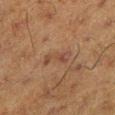Acquisition and patient details: The subject is a male aged 58–62. The lesion-visualizer software estimated a mean CIELAB color near L≈35 a*≈16 b*≈24, roughly 5 lightness units darker than nearby skin, and a normalized border contrast of about 5. It also reported a border-irregularity rating of about 4.5/10, a within-lesion color-variation index near 2.5/10, and radial color variation of about 0.5. The lesion is located on the leg. Measured at roughly 3.5 mm in maximum diameter. The tile uses cross-polarized illumination. Cropped from a whole-body photographic skin survey; the tile spans about 15 mm.